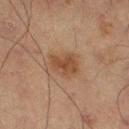This lesion was catalogued during total-body skin photography and was not selected for biopsy.
The lesion-visualizer software estimated a lesion color around L≈37 a*≈16 b*≈27 in CIELAB and a lesion-to-skin contrast of about 7 (normalized; higher = more distinct). And it measured border irregularity of about 2.5 on a 0–10 scale and radial color variation of about 1. And it measured lesion-presence confidence of about 100/100.
A roughly 15 mm field-of-view crop from a total-body skin photograph.
Imaged with cross-polarized lighting.
Located on the left thigh.
A male subject aged 63 to 67.
Approximately 3.5 mm at its widest.